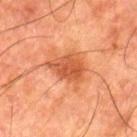About 5 mm across.
This is a cross-polarized tile.
The patient is a male aged approximately 80.
A lesion tile, about 15 mm wide, cut from a 3D total-body photograph.
Automated tile analysis of the lesion measured an eccentricity of roughly 0.75 and a symmetry-axis asymmetry near 0.3. And it measured an average lesion color of about L≈44 a*≈26 b*≈33 (CIELAB), roughly 10 lightness units darker than nearby skin, and a normalized border contrast of about 7.5. The software also gave a border-irregularity rating of about 3.5/10. The analysis additionally found a classifier nevus-likeness of about 75/100 and a detector confidence of about 100 out of 100 that the crop contains a lesion.
The lesion is on the left thigh.